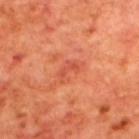biopsy status = total-body-photography surveillance lesion; no biopsy
image = 15 mm crop, total-body photography
subject = male, aged approximately 70
illumination = cross-polarized
location = the upper back